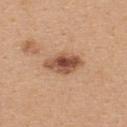location — the upper back | subject — female, roughly 25 years of age | imaging modality — total-body-photography crop, ~15 mm field of view.